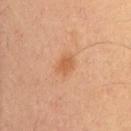Impression:
Imaged during a routine full-body skin examination; the lesion was not biopsied and no histopathology is available.
Image and clinical context:
Measured at roughly 2.5 mm in maximum diameter. Imaged with cross-polarized lighting. A male patient, in their 60s. The lesion is located on the abdomen. This image is a 15 mm lesion crop taken from a total-body photograph.